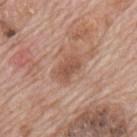Clinical impression: The lesion was photographed on a routine skin check and not biopsied; there is no pathology result. Acquisition and patient details: Located on the mid back. The patient is a male roughly 70 years of age. Cropped from a total-body skin-imaging series; the visible field is about 15 mm.